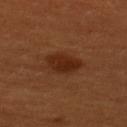Notes:
• biopsy status: total-body-photography surveillance lesion; no biopsy
• size: ~4.5 mm (longest diameter)
• image source: 15 mm crop, total-body photography
• automated lesion analysis: a lesion area of about 9.5 mm², an eccentricity of roughly 0.75, and a symmetry-axis asymmetry near 0.2; border irregularity of about 2 on a 0–10 scale and peripheral color asymmetry of about 1; an automated nevus-likeness rating near 100 out of 100
• subject: female, aged around 50